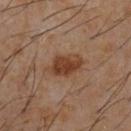follow-up: total-body-photography surveillance lesion; no biopsy | diameter: about 4.5 mm | imaging modality: total-body-photography crop, ~15 mm field of view | location: the chest | patient: male, roughly 60 years of age | TBP lesion metrics: a lesion area of about 9 mm² and a symmetry-axis asymmetry near 0.2; a border-irregularity index near 2.5/10, internal color variation of about 4 on a 0–10 scale, and a peripheral color-asymmetry measure near 1.5.